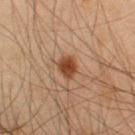The lesion was photographed on a routine skin check and not biopsied; there is no pathology result.
The subject is a male approximately 45 years of age.
From the left upper arm.
Measured at roughly 2.5 mm in maximum diameter.
A region of skin cropped from a whole-body photographic capture, roughly 15 mm wide.